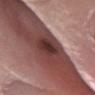biopsy status = catalogued during a skin exam; not biopsied | automated metrics = a lesion area of about 7 mm² and an eccentricity of roughly 0.85; an average lesion color of about L≈33 a*≈23 b*≈20 (CIELAB), about 14 CIELAB-L* units darker than the surrounding skin, and a normalized border contrast of about 11.5 | image = ~15 mm crop, total-body skin-cancer survey | lighting = white-light illumination | subject = male, aged around 60 | location = the right lower leg | diameter = ≈4 mm.